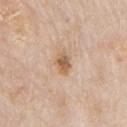patient=male, about 75 years old | image source=~15 mm tile from a whole-body skin photo | anatomic site=the front of the torso.